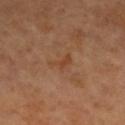Acquisition and patient details: A female patient, roughly 55 years of age. Imaged with cross-polarized lighting. On the right lower leg. A 15 mm close-up extracted from a 3D total-body photography capture.